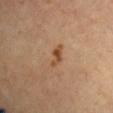No biopsy was performed on this lesion — it was imaged during a full skin examination and was not determined to be concerning. The subject is a female in their mid-60s. This is a cross-polarized tile. Approximately 3 mm at its widest. The lesion is located on the chest. A 15 mm close-up tile from a total-body photography series done for melanoma screening.